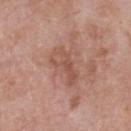workup: total-body-photography surveillance lesion; no biopsy
image-analysis metrics: a footprint of about 8 mm², an outline eccentricity of about 0.9 (0 = round, 1 = elongated), and a shape-asymmetry score of about 0.45 (0 = symmetric); a nevus-likeness score of about 0/100 and a detector confidence of about 100 out of 100 that the crop contains a lesion
patient: male, aged approximately 55
imaging modality: ~15 mm tile from a whole-body skin photo
lesion size: ~4.5 mm (longest diameter)
body site: the front of the torso